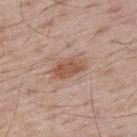notes: total-body-photography surveillance lesion; no biopsy | tile lighting: white-light illumination | lesion diameter: ≈4.5 mm | automated lesion analysis: an average lesion color of about L≈55 a*≈20 b*≈29 (CIELAB), roughly 10 lightness units darker than nearby skin, and a normalized border contrast of about 7.5; a border-irregularity rating of about 2.5/10 and a color-variation rating of about 3/10; an automated nevus-likeness rating near 70 out of 100 and lesion-presence confidence of about 100/100 | acquisition: ~15 mm tile from a whole-body skin photo | patient: male, about 65 years old | site: the upper back.